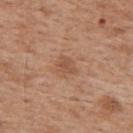– workup · imaged on a skin check; not biopsied
– tile lighting · white-light illumination
– acquisition · 15 mm crop, total-body photography
– patient · male, in their 60s
– body site · the back
– diameter · about 2.5 mm